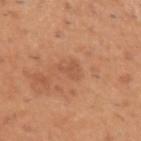biopsy status: no biopsy performed (imaged during a skin exam)
illumination: white-light
site: the left upper arm
subject: male, about 40 years old
lesion diameter: ~2.5 mm (longest diameter)
image: ~15 mm tile from a whole-body skin photo